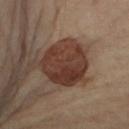* follow-up: total-body-photography surveillance lesion; no biopsy
* TBP lesion metrics: an area of roughly 22 mm², an eccentricity of roughly 0.4, and a symmetry-axis asymmetry near 0.15; a mean CIELAB color near L≈35 a*≈19 b*≈24 and a lesion-to-skin contrast of about 10 (normalized; higher = more distinct)
* illumination: cross-polarized illumination
* acquisition: ~15 mm tile from a whole-body skin photo
* subject: female, aged around 60
* site: the right upper arm
* diameter: about 5.5 mm